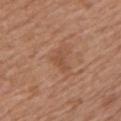Q: Is there a histopathology result?
A: imaged on a skin check; not biopsied
Q: What kind of image is this?
A: total-body-photography crop, ~15 mm field of view
Q: Lesion location?
A: the left upper arm
Q: Who is the patient?
A: male, in their mid-60s